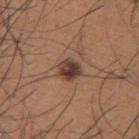The patient is a male in their mid-30s. The total-body-photography lesion software estimated a lesion color around L≈40 a*≈19 b*≈25 in CIELAB and a lesion–skin lightness drop of about 13. Captured under white-light illumination. The recorded lesion diameter is about 3 mm. A region of skin cropped from a whole-body photographic capture, roughly 15 mm wide. From the upper back.